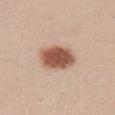• tile lighting — white-light illumination
• subject — female, aged 23 to 27
• acquisition — 15 mm crop, total-body photography
• TBP lesion metrics — a shape-asymmetry score of about 0.15 (0 = symmetric); roughly 17 lightness units darker than nearby skin and a normalized border contrast of about 11; border irregularity of about 1.5 on a 0–10 scale and peripheral color asymmetry of about 1
• diameter — about 5 mm
• location — the left thigh